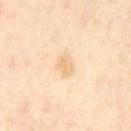Image and clinical context: About 2.5 mm across. The patient is roughly 55 years of age. The lesion is on the chest. A lesion tile, about 15 mm wide, cut from a 3D total-body photograph. The tile uses cross-polarized illumination.